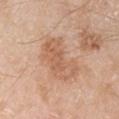Context:
The lesion's longest dimension is about 6.5 mm. The subject is a male about 80 years old. A close-up tile cropped from a whole-body skin photograph, about 15 mm across. Captured under white-light illumination. An algorithmic analysis of the crop reported a shape eccentricity near 0.85. The software also gave a border-irregularity rating of about 4/10, a within-lesion color-variation index near 3.5/10, and peripheral color asymmetry of about 1. And it measured an automated nevus-likeness rating near 0 out of 100 and a lesion-detection confidence of about 100/100. Located on the right upper arm.